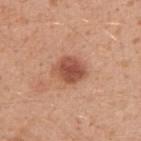notes = total-body-photography surveillance lesion; no biopsy
anatomic site = the right upper arm
image source = total-body-photography crop, ~15 mm field of view
patient = male, roughly 30 years of age